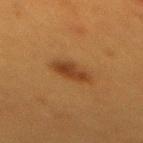Impression: Captured during whole-body skin photography for melanoma surveillance; the lesion was not biopsied. Image and clinical context: From the mid back. Imaged with cross-polarized lighting. The patient is a female aged around 40. Cropped from a whole-body photographic skin survey; the tile spans about 15 mm. The recorded lesion diameter is about 4.5 mm.